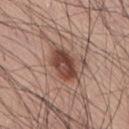notes: catalogued during a skin exam; not biopsied